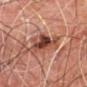This lesion was catalogued during total-body skin photography and was not selected for biopsy. On the right leg. A 15 mm close-up tile from a total-body photography series done for melanoma screening. A male subject in their 60s.